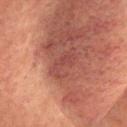follow-up: no biopsy performed (imaged during a skin exam) | image: 15 mm crop, total-body photography | site: the head or neck | illumination: cross-polarized illumination | subject: female, in their 60s.